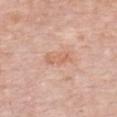lesion size = ≈3.5 mm
imaging modality = ~15 mm crop, total-body skin-cancer survey
image-analysis metrics = a normalized border contrast of about 5.5; a border-irregularity rating of about 3.5/10, a within-lesion color-variation index near 2.5/10, and radial color variation of about 0.5
lighting = white-light illumination
subject = female, aged around 65
anatomic site = the front of the torso